A male patient, aged around 70. Cropped from a total-body skin-imaging series; the visible field is about 15 mm. The lesion is located on the chest: 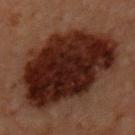Histopathology of the biopsied lesion showed a dysplastic (Clark) nevus — a benign lesion.